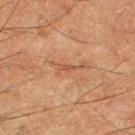Findings:
– biopsy status: total-body-photography surveillance lesion; no biopsy